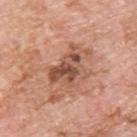workup: no biopsy performed (imaged during a skin exam) | image: 15 mm crop, total-body photography | patient: male, in their 60s | location: the upper back.